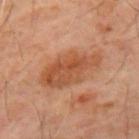The lesion was photographed on a routine skin check and not biopsied; there is no pathology result. Approximately 7 mm at its widest. The patient is a male in their 60s. Imaged with cross-polarized lighting. From the mid back. An algorithmic analysis of the crop reported a within-lesion color-variation index near 4.5/10 and a peripheral color-asymmetry measure near 1.5. A 15 mm crop from a total-body photograph taken for skin-cancer surveillance.